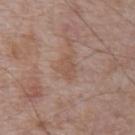| field | value |
|---|---|
| workup | no biopsy performed (imaged during a skin exam) |
| lesion size | ≈3 mm |
| lighting | white-light illumination |
| image source | total-body-photography crop, ~15 mm field of view |
| location | the abdomen |
| patient | male, about 70 years old |